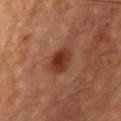Recorded during total-body skin imaging; not selected for excision or biopsy. From the chest. Imaged with cross-polarized lighting. Cropped from a total-body skin-imaging series; the visible field is about 15 mm. Longest diameter approximately 4 mm. Automated tile analysis of the lesion measured a mean CIELAB color near L≈36 a*≈25 b*≈30, about 11 CIELAB-L* units darker than the surrounding skin, and a normalized border contrast of about 9. It also reported a border-irregularity rating of about 2/10, a within-lesion color-variation index near 4/10, and peripheral color asymmetry of about 1.5. The analysis additionally found an automated nevus-likeness rating near 95 out of 100 and a detector confidence of about 100 out of 100 that the crop contains a lesion. A male patient, in their mid- to late 50s.